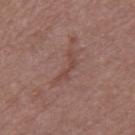The lesion was photographed on a routine skin check and not biopsied; there is no pathology result. A 15 mm close-up tile from a total-body photography series done for melanoma screening. An algorithmic analysis of the crop reported a shape eccentricity near 0.95 and a symmetry-axis asymmetry near 0.7. And it measured a color-variation rating of about 0/10. Measured at roughly 4 mm in maximum diameter. The lesion is on the right thigh. A male subject aged approximately 75.